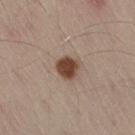Q: Is there a histopathology result?
A: imaged on a skin check; not biopsied
Q: Patient demographics?
A: male, about 55 years old
Q: What kind of image is this?
A: ~15 mm crop, total-body skin-cancer survey
Q: Lesion location?
A: the right thigh